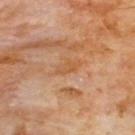Assessment:
The lesion was tiled from a total-body skin photograph and was not biopsied.
Clinical summary:
A male subject aged 58–62. Located on the chest. A lesion tile, about 15 mm wide, cut from a 3D total-body photograph. Captured under cross-polarized illumination.